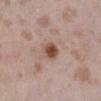Case summary:
– follow-up — imaged on a skin check; not biopsied
– subject — female, roughly 25 years of age
– imaging modality — ~15 mm tile from a whole-body skin photo
– body site — the right lower leg
– lesion diameter — ~2.5 mm (longest diameter)
– TBP lesion metrics — internal color variation of about 3.5 on a 0–10 scale and radial color variation of about 1